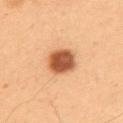follow-up = total-body-photography surveillance lesion; no biopsy
image source = 15 mm crop, total-body photography
subject = male, aged 53 to 57
body site = the upper back